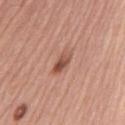Clinical impression:
No biopsy was performed on this lesion — it was imaged during a full skin examination and was not determined to be concerning.
Context:
The patient is a female roughly 60 years of age. The recorded lesion diameter is about 3 mm. A close-up tile cropped from a whole-body skin photograph, about 15 mm across. On the left upper arm.